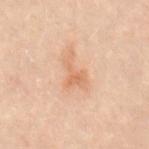Clinical impression: Imaged during a routine full-body skin examination; the lesion was not biopsied and no histopathology is available. Background: The lesion is located on the back. Imaged with cross-polarized lighting. A female patient, roughly 55 years of age. A roughly 15 mm field-of-view crop from a total-body skin photograph. The total-body-photography lesion software estimated an area of roughly 7.5 mm² and a symmetry-axis asymmetry near 0.55. The software also gave a nevus-likeness score of about 0/100.